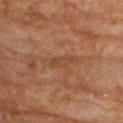follow-up = total-body-photography surveillance lesion; no biopsy
patient = female, aged around 80
site = the upper back
image source = ~15 mm tile from a whole-body skin photo
lesion size = ≈3.5 mm